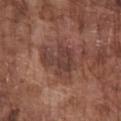<case>
<image>
  <source>total-body photography crop</source>
  <field_of_view_mm>15</field_of_view_mm>
</image>
<patient>
  <sex>male</sex>
  <age_approx>75</age_approx>
</patient>
<site>chest</site>
</case>